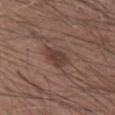Recorded during total-body skin imaging; not selected for excision or biopsy.
Captured under white-light illumination.
A 15 mm close-up extracted from a 3D total-body photography capture.
Located on the arm.
Longest diameter approximately 3.5 mm.
The patient is a male in their mid-50s.